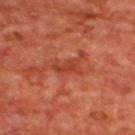This lesion was catalogued during total-body skin photography and was not selected for biopsy. A male patient approximately 65 years of age. A 15 mm close-up extracted from a 3D total-body photography capture. From the upper back.